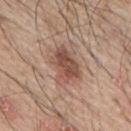This lesion was catalogued during total-body skin photography and was not selected for biopsy. The tile uses white-light illumination. A male subject, in their mid- to late 60s. Located on the back. A region of skin cropped from a whole-body photographic capture, roughly 15 mm wide. Approximately 4.5 mm at its widest.